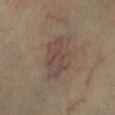Q: Was this lesion biopsied?
A: no biopsy performed (imaged during a skin exam)
Q: What is the imaging modality?
A: 15 mm crop, total-body photography
Q: Lesion location?
A: the right lower leg
Q: Illumination type?
A: cross-polarized illumination
Q: What are the patient's age and sex?
A: male, aged 53 to 57